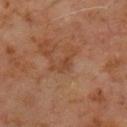A male patient aged around 60. A 15 mm close-up tile from a total-body photography series done for melanoma screening. An algorithmic analysis of the crop reported a border-irregularity index near 8/10, a color-variation rating of about 1.5/10, and a peripheral color-asymmetry measure near 0.5. The analysis additionally found a classifier nevus-likeness of about 0/100. The lesion is on the front of the torso. The recorded lesion diameter is about 3.5 mm. This is a cross-polarized tile.